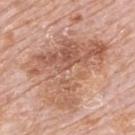{
  "patient": {
    "sex": "male",
    "age_approx": 80
  },
  "site": "upper back",
  "automated_metrics": {
    "area_mm2_approx": 38.0,
    "shape_asymmetry": 0.55,
    "cielab_L": 58,
    "cielab_a": 22,
    "cielab_b": 30,
    "vs_skin_darker_L": 10.0,
    "vs_skin_contrast_norm": 7.0
  },
  "lighting": "white-light",
  "lesion_size": {
    "long_diameter_mm_approx": 9.0
  },
  "image": {
    "source": "total-body photography crop",
    "field_of_view_mm": 15
  }
}